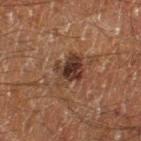{
  "biopsy_status": "not biopsied; imaged during a skin examination",
  "lighting": "cross-polarized",
  "image": {
    "source": "total-body photography crop",
    "field_of_view_mm": 15
  },
  "site": "left thigh",
  "patient": {
    "sex": "male",
    "age_approx": 60
  },
  "automated_metrics": {
    "cielab_L": 26,
    "cielab_a": 15,
    "cielab_b": 21,
    "vs_skin_contrast_norm": 10.0
  },
  "lesion_size": {
    "long_diameter_mm_approx": 4.0
  }
}